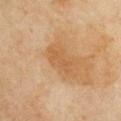Assessment: The lesion was tiled from a total-body skin photograph and was not biopsied. Acquisition and patient details: This image is a 15 mm lesion crop taken from a total-body photograph. The subject is a female aged 68–72. Located on the front of the torso.